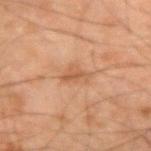{"biopsy_status": "not biopsied; imaged during a skin examination", "automated_metrics": {"area_mm2_approx": 4.0, "eccentricity": 0.8, "shape_asymmetry": 0.45, "cielab_L": 48, "cielab_a": 20, "cielab_b": 31, "vs_skin_darker_L": 7.0, "border_irregularity_0_10": 4.5, "peripheral_color_asymmetry": 1.0}, "patient": {"sex": "male", "age_approx": 65}, "site": "right forearm", "image": {"source": "total-body photography crop", "field_of_view_mm": 15}}